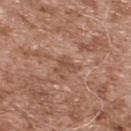  biopsy_status: not biopsied; imaged during a skin examination
  site: upper back
  automated_metrics:
    cielab_L: 50
    cielab_a: 21
    cielab_b: 30
    vs_skin_darker_L: 7.0
    vs_skin_contrast_norm: 5.5
    nevus_likeness_0_100: 0
  lighting: white-light
  patient:
    sex: male
    age_approx: 55
  image:
    source: total-body photography crop
    field_of_view_mm: 15